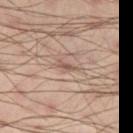Approximately 2.5 mm at its widest. The tile uses cross-polarized illumination. The subject is a male aged approximately 40. A close-up tile cropped from a whole-body skin photograph, about 15 mm across. The lesion is on the right thigh. The lesion-visualizer software estimated an average lesion color of about L≈53 a*≈17 b*≈24 (CIELAB).